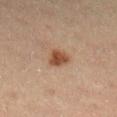The lesion was tiled from a total-body skin photograph and was not biopsied. The subject is a female in their 30s. Imaged with cross-polarized lighting. A roughly 15 mm field-of-view crop from a total-body skin photograph. The lesion is located on the right lower leg. Automated image analysis of the tile measured a border-irregularity index near 2/10, a within-lesion color-variation index near 3/10, and a peripheral color-asymmetry measure near 1. The software also gave a classifier nevus-likeness of about 95/100. About 3 mm across.